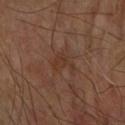follow-up — catalogued during a skin exam; not biopsied
acquisition — ~15 mm tile from a whole-body skin photo
location — the arm
subject — male, approximately 70 years of age